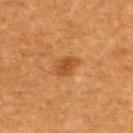{
  "biopsy_status": "not biopsied; imaged during a skin examination",
  "site": "upper back",
  "automated_metrics": {
    "eccentricity": 0.7,
    "vs_skin_contrast_norm": 7.5
  },
  "lesion_size": {
    "long_diameter_mm_approx": 2.5
  },
  "lighting": "cross-polarized",
  "patient": {
    "sex": "male",
    "age_approx": 60
  },
  "image": {
    "source": "total-body photography crop",
    "field_of_view_mm": 15
  }
}